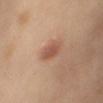<lesion>
<biopsy_status>not biopsied; imaged during a skin examination</biopsy_status>
<lesion_size>
  <long_diameter_mm_approx>3.5</long_diameter_mm_approx>
</lesion_size>
<site>right upper arm</site>
<lighting>cross-polarized</lighting>
<image>
  <source>total-body photography crop</source>
  <field_of_view_mm>15</field_of_view_mm>
</image>
<automated_metrics>
  <peripheral_color_asymmetry>1.0</peripheral_color_asymmetry>
</automated_metrics>
<patient>
  <sex>female</sex>
  <age_approx>55</age_approx>
</patient>
</lesion>